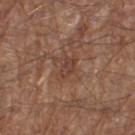Clinical impression:
The lesion was photographed on a routine skin check and not biopsied; there is no pathology result.
Image and clinical context:
A 15 mm crop from a total-body photograph taken for skin-cancer surveillance. This is a white-light tile. From the left thigh. Approximately 2.5 mm at its widest. A male patient, roughly 65 years of age.